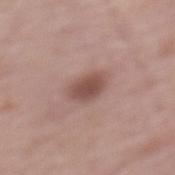Q: Is there a histopathology result?
A: imaged on a skin check; not biopsied
Q: What lighting was used for the tile?
A: white-light
Q: Who is the patient?
A: female, roughly 65 years of age
Q: What is the anatomic site?
A: the mid back
Q: What did automated image analysis measure?
A: an average lesion color of about L≈50 a*≈20 b*≈23 (CIELAB), roughly 11 lightness units darker than nearby skin, and a normalized border contrast of about 8
Q: How was this image acquired?
A: total-body-photography crop, ~15 mm field of view